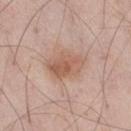The lesion was tiled from a total-body skin photograph and was not biopsied. This image is a 15 mm lesion crop taken from a total-body photograph. A male patient aged around 55. The recorded lesion diameter is about 5 mm. Located on the right thigh. This is a white-light tile.